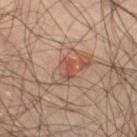| key | value |
|---|---|
| workup | no biopsy performed (imaged during a skin exam) |
| anatomic site | the right thigh |
| lesion size | ≈2.5 mm |
| subject | male, aged 63 to 67 |
| lighting | cross-polarized |
| acquisition | ~15 mm crop, total-body skin-cancer survey |